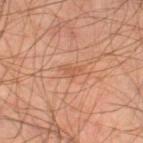Impression:
The lesion was tiled from a total-body skin photograph and was not biopsied.
Context:
An algorithmic analysis of the crop reported an outline eccentricity of about 0.8 (0 = round, 1 = elongated) and a shape-asymmetry score of about 0.45 (0 = symmetric). The analysis additionally found border irregularity of about 4.5 on a 0–10 scale and radial color variation of about 0.5. The recorded lesion diameter is about 2.5 mm. The lesion is on the right lower leg. A male patient, roughly 45 years of age. This image is a 15 mm lesion crop taken from a total-body photograph. Imaged with cross-polarized lighting.